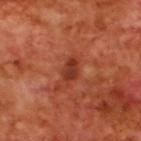notes: imaged on a skin check; not biopsied
anatomic site: the upper back
acquisition: 15 mm crop, total-body photography
patient: male, in their 70s
diameter: ~3.5 mm (longest diameter)
tile lighting: cross-polarized
TBP lesion metrics: a border-irregularity rating of about 3/10, a color-variation rating of about 3.5/10, and radial color variation of about 1; a nevus-likeness score of about 40/100